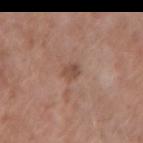<case>
<patient>
  <sex>female</sex>
  <age_approx>65</age_approx>
</patient>
<image>
  <source>total-body photography crop</source>
  <field_of_view_mm>15</field_of_view_mm>
</image>
<site>left forearm</site>
</case>